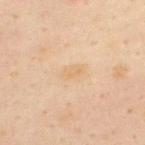Part of a total-body skin-imaging series; this lesion was reviewed on a skin check and was not flagged for biopsy.
Automated tile analysis of the lesion measured roughly 5 lightness units darker than nearby skin. The software also gave border irregularity of about 3.5 on a 0–10 scale, internal color variation of about 1 on a 0–10 scale, and a peripheral color-asymmetry measure near 0.5. The analysis additionally found a classifier nevus-likeness of about 0/100 and a detector confidence of about 100 out of 100 that the crop contains a lesion.
From the upper back.
A male subject, aged 48–52.
A region of skin cropped from a whole-body photographic capture, roughly 15 mm wide.
The tile uses cross-polarized illumination.
Approximately 2.5 mm at its widest.